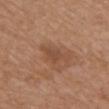Clinical impression: Part of a total-body skin-imaging series; this lesion was reviewed on a skin check and was not flagged for biopsy. Image and clinical context: Located on the chest. A 15 mm crop from a total-body photograph taken for skin-cancer surveillance. This is a white-light tile. The patient is a female aged 68 to 72. Automated image analysis of the tile measured roughly 8 lightness units darker than nearby skin. The software also gave a border-irregularity rating of about 5/10, a color-variation rating of about 1/10, and peripheral color asymmetry of about 0.5. About 4 mm across.